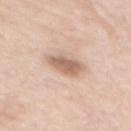Q: Was a biopsy performed?
A: no biopsy performed (imaged during a skin exam)
Q: What is the anatomic site?
A: the back
Q: Patient demographics?
A: female, about 70 years old
Q: Lesion size?
A: ≈3.5 mm
Q: What is the imaging modality?
A: total-body-photography crop, ~15 mm field of view
Q: How was the tile lit?
A: white-light
Q: What did automated image analysis measure?
A: an area of roughly 6.5 mm² and a shape-asymmetry score of about 0.25 (0 = symmetric); an average lesion color of about L≈62 a*≈19 b*≈28 (CIELAB), a lesion–skin lightness drop of about 13, and a lesion-to-skin contrast of about 8 (normalized; higher = more distinct); a within-lesion color-variation index near 2.5/10 and radial color variation of about 1